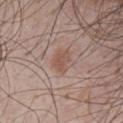Part of a total-body skin-imaging series; this lesion was reviewed on a skin check and was not flagged for biopsy. A male subject aged 48 to 52. The lesion is located on the front of the torso. This image is a 15 mm lesion crop taken from a total-body photograph.